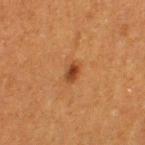On the leg. Cropped from a whole-body photographic skin survey; the tile spans about 15 mm. A female subject, in their 40s.